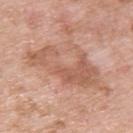The tile uses white-light illumination.
A region of skin cropped from a whole-body photographic capture, roughly 15 mm wide.
On the upper back.
Longest diameter approximately 9 mm.
The patient is a male aged 48–52.
Automated image analysis of the tile measured an area of roughly 24 mm² and an eccentricity of roughly 0.9. The software also gave an average lesion color of about L≈59 a*≈22 b*≈30 (CIELAB) and roughly 9 lightness units darker than nearby skin. The analysis additionally found an automated nevus-likeness rating near 0 out of 100.